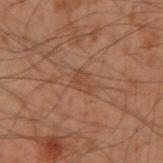The lesion was tiled from a total-body skin photograph and was not biopsied. Approximately 3 mm at its widest. A 15 mm crop from a total-body photograph taken for skin-cancer surveillance. The lesion is on the arm. A male subject aged around 50. The tile uses cross-polarized illumination.